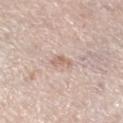The lesion was photographed on a routine skin check and not biopsied; there is no pathology result. Approximately 2.5 mm at its widest. The lesion-visualizer software estimated an outline eccentricity of about 0.85 (0 = round, 1 = elongated) and two-axis asymmetry of about 0.45. The analysis additionally found an automated nevus-likeness rating near 0 out of 100 and a detector confidence of about 90 out of 100 that the crop contains a lesion. A female patient approximately 65 years of age. Captured under white-light illumination. The lesion is on the left lower leg. A lesion tile, about 15 mm wide, cut from a 3D total-body photograph.